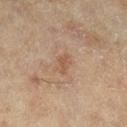This lesion was catalogued during total-body skin photography and was not selected for biopsy.
A lesion tile, about 15 mm wide, cut from a 3D total-body photograph.
The subject is a female aged around 60.
This is a cross-polarized tile.
The lesion is located on the right lower leg.